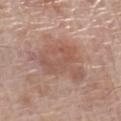Q: Was this lesion biopsied?
A: catalogued during a skin exam; not biopsied
Q: What is the lesion's diameter?
A: ~7 mm (longest diameter)
Q: What are the patient's age and sex?
A: male, aged around 70
Q: What is the anatomic site?
A: the left lower leg
Q: How was this image acquired?
A: ~15 mm tile from a whole-body skin photo
Q: How was the tile lit?
A: white-light